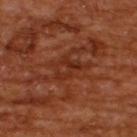Recorded during total-body skin imaging; not selected for excision or biopsy. A 15 mm close-up tile from a total-body photography series done for melanoma screening. A male subject, aged 63–67. On the upper back.